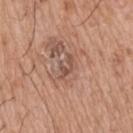Assessment: This lesion was catalogued during total-body skin photography and was not selected for biopsy. Image and clinical context: Approximately 3 mm at its widest. The lesion is on the head or neck. A lesion tile, about 15 mm wide, cut from a 3D total-body photograph. The patient is a male about 75 years old. Automated tile analysis of the lesion measured a border-irregularity index near 6.5/10, a color-variation rating of about 0/10, and peripheral color asymmetry of about 0. The software also gave a classifier nevus-likeness of about 0/100 and lesion-presence confidence of about 95/100.